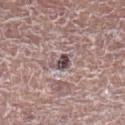* workup · no biopsy performed (imaged during a skin exam)
* body site · the leg
* image source · 15 mm crop, total-body photography
* patient · male, aged around 80